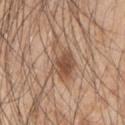Findings:
* workup: no biopsy performed (imaged during a skin exam)
* lesion size: about 5 mm
* image-analysis metrics: a border-irregularity rating of about 4/10, internal color variation of about 6.5 on a 0–10 scale, and a peripheral color-asymmetry measure near 2; a lesion-detection confidence of about 100/100
* anatomic site: the left upper arm
* imaging modality: ~15 mm crop, total-body skin-cancer survey
* lighting: white-light
* patient: male, approximately 50 years of age